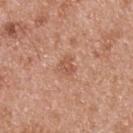Q: Is there a histopathology result?
A: catalogued during a skin exam; not biopsied
Q: What is the imaging modality?
A: total-body-photography crop, ~15 mm field of view
Q: What are the patient's age and sex?
A: male, aged 53 to 57
Q: Where on the body is the lesion?
A: the upper back